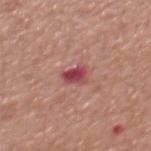notes — no biopsy performed (imaged during a skin exam)
body site — the mid back
imaging modality — total-body-photography crop, ~15 mm field of view
image-analysis metrics — a lesion color around L≈47 a*≈32 b*≈21 in CIELAB, roughly 13 lightness units darker than nearby skin, and a normalized lesion–skin contrast near 9.5; a border-irregularity rating of about 3/10 and a color-variation rating of about 5.5/10; a classifier nevus-likeness of about 0/100 and a detector confidence of about 100 out of 100 that the crop contains a lesion
subject — male, approximately 65 years of age
size — ~3 mm (longest diameter)